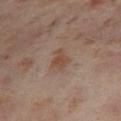<lesion>
<biopsy_status>not biopsied; imaged during a skin examination</biopsy_status>
<patient>
  <sex>female</sex>
  <age_approx>55</age_approx>
</patient>
<image>
  <source>total-body photography crop</source>
  <field_of_view_mm>15</field_of_view_mm>
</image>
<automated_metrics>
  <cielab_L>47</cielab_L>
  <cielab_a>18</cielab_a>
  <cielab_b>28</cielab_b>
  <vs_skin_darker_L>8.0</vs_skin_darker_L>
  <vs_skin_contrast_norm>7.0</vs_skin_contrast_norm>
  <peripheral_color_asymmetry>0.5</peripheral_color_asymmetry>
</automated_metrics>
<lighting>cross-polarized</lighting>
<site>right thigh</site>
</lesion>